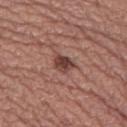notes = imaged on a skin check; not biopsied
location = the left thigh
automated lesion analysis = an average lesion color of about L≈41 a*≈22 b*≈23 (CIELAB), roughly 13 lightness units darker than nearby skin, and a normalized lesion–skin contrast near 10; border irregularity of about 2.5 on a 0–10 scale, internal color variation of about 3.5 on a 0–10 scale, and peripheral color asymmetry of about 1; an automated nevus-likeness rating near 50 out of 100 and a detector confidence of about 100 out of 100 that the crop contains a lesion
size = ~2.5 mm (longest diameter)
image = ~15 mm crop, total-body skin-cancer survey
patient = female, roughly 55 years of age
illumination = white-light